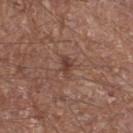Case summary:
– workup — no biopsy performed (imaged during a skin exam)
– subject — male, aged approximately 65
– anatomic site — the right thigh
– illumination — white-light
– size — about 2.5 mm
– image source — ~15 mm crop, total-body skin-cancer survey